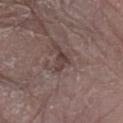The lesion was tiled from a total-body skin photograph and was not biopsied. A male patient aged 78 to 82. The lesion is on the right arm. A roughly 15 mm field-of-view crop from a total-body skin photograph.